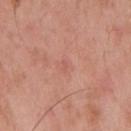follow-up: total-body-photography surveillance lesion; no biopsy | imaging modality: ~15 mm tile from a whole-body skin photo | location: the mid back | subject: male, roughly 55 years of age.